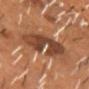The lesion was photographed on a routine skin check and not biopsied; there is no pathology result. Automated tile analysis of the lesion measured a footprint of about 18 mm², an eccentricity of roughly 0.85, and a shape-asymmetry score of about 0.25 (0 = symmetric). It also reported roughly 12 lightness units darker than nearby skin. And it measured a border-irregularity rating of about 5.5/10 and peripheral color asymmetry of about 1. And it measured an automated nevus-likeness rating near 0 out of 100. This is a cross-polarized tile. A male subject, approximately 55 years of age. The lesion is located on the head or neck. A region of skin cropped from a whole-body photographic capture, roughly 15 mm wide.